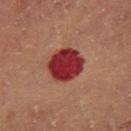Clinical impression: Recorded during total-body skin imaging; not selected for excision or biopsy.